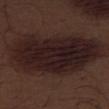Findings:
- workup: no biopsy performed (imaged during a skin exam)
- TBP lesion metrics: an average lesion color of about L≈19 a*≈15 b*≈15 (CIELAB), a lesion–skin lightness drop of about 9, and a normalized border contrast of about 12.5; a color-variation rating of about 5/10; an automated nevus-likeness rating near 60 out of 100 and a lesion-detection confidence of about 80/100
- tile lighting: white-light illumination
- image source: total-body-photography crop, ~15 mm field of view
- location: the right thigh
- lesion size: about 13 mm
- patient: male, aged 68 to 72